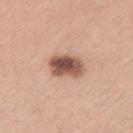This lesion was catalogued during total-body skin photography and was not selected for biopsy. A 15 mm close-up extracted from a 3D total-body photography capture. From the right thigh. A female subject, aged 58–62.